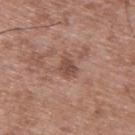| field | value |
|---|---|
| follow-up | total-body-photography surveillance lesion; no biopsy |
| patient | male, approximately 50 years of age |
| automated metrics | an eccentricity of roughly 0.6 and a symmetry-axis asymmetry near 0.3; about 10 CIELAB-L* units darker than the surrounding skin and a normalized border contrast of about 7; a border-irregularity rating of about 2.5/10 and a within-lesion color-variation index near 2.5/10; an automated nevus-likeness rating near 0 out of 100 and lesion-presence confidence of about 100/100 |
| acquisition | ~15 mm crop, total-body skin-cancer survey |
| tile lighting | white-light |
| diameter | ~2.5 mm (longest diameter) |
| site | the upper back |